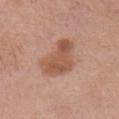This lesion was catalogued during total-body skin photography and was not selected for biopsy.
Cropped from a total-body skin-imaging series; the visible field is about 15 mm.
The lesion is located on the chest.
A female patient aged 63–67.
Measured at roughly 5.5 mm in maximum diameter.
This is a white-light tile.